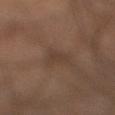<tbp_lesion>
  <biopsy_status>not biopsied; imaged during a skin examination</biopsy_status>
  <lighting>cross-polarized</lighting>
  <lesion_size>
    <long_diameter_mm_approx>3.0</long_diameter_mm_approx>
  </lesion_size>
  <patient>
    <sex>male</sex>
    <age_approx>60</age_approx>
  </patient>
  <image>
    <source>total-body photography crop</source>
    <field_of_view_mm>15</field_of_view_mm>
  </image>
  <site>right lower leg</site>
</tbp_lesion>